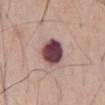biopsy_status: not biopsied; imaged during a skin examination
site: chest
lighting: white-light
image:
  source: total-body photography crop
  field_of_view_mm: 15
patient:
  sex: male
  age_approx: 70